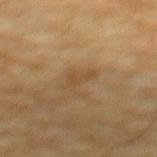The lesion was tiled from a total-body skin photograph and was not biopsied. On the mid back. The lesion's longest dimension is about 3 mm. A male subject, roughly 70 years of age. Automated tile analysis of the lesion measured border irregularity of about 5 on a 0–10 scale and peripheral color asymmetry of about 0. It also reported an automated nevus-likeness rating near 0 out of 100 and lesion-presence confidence of about 100/100. The tile uses cross-polarized illumination. This image is a 15 mm lesion crop taken from a total-body photograph.